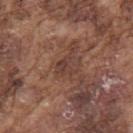{"biopsy_status": "not biopsied; imaged during a skin examination", "image": {"source": "total-body photography crop", "field_of_view_mm": 15}, "site": "arm", "automated_metrics": {"area_mm2_approx": 4.5, "eccentricity": 0.55, "shape_asymmetry": 0.35, "nevus_likeness_0_100": 0, "lesion_detection_confidence_0_100": 85}, "patient": {"sex": "male", "age_approx": 75}, "lighting": "white-light"}